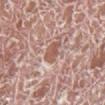A roughly 15 mm field-of-view crop from a total-body skin photograph. An algorithmic analysis of the crop reported an area of roughly 4.5 mm² and a shape-asymmetry score of about 0.3 (0 = symmetric). The analysis additionally found a lesion color around L≈56 a*≈22 b*≈27 in CIELAB, about 10 CIELAB-L* units darker than the surrounding skin, and a normalized border contrast of about 7. It also reported a border-irregularity index near 3/10 and a peripheral color-asymmetry measure near 0.5. A male subject in their mid- to late 70s. The tile uses white-light illumination. About 3 mm across. On the right lower leg.